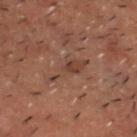follow-up = catalogued during a skin exam; not biopsied | size = about 5 mm | anatomic site = the head or neck | image-analysis metrics = a lesion color around L≈31 a*≈15 b*≈21 in CIELAB, about 5 CIELAB-L* units darker than the surrounding skin, and a normalized lesion–skin contrast near 5.5; border irregularity of about 5.5 on a 0–10 scale and a color-variation rating of about 2.5/10; an automated nevus-likeness rating near 0 out of 100 | image = total-body-photography crop, ~15 mm field of view | lighting = cross-polarized | patient = male, aged 48 to 52.